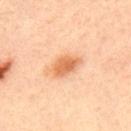Notes:
* workup: imaged on a skin check; not biopsied
* patient: male, aged 33–37
* image source: ~15 mm tile from a whole-body skin photo
* lighting: cross-polarized illumination
* lesion diameter: ≈4 mm
* site: the upper back
* image-analysis metrics: an average lesion color of about L≈66 a*≈26 b*≈40 (CIELAB), roughly 12 lightness units darker than nearby skin, and a lesion-to-skin contrast of about 8 (normalized; higher = more distinct); a border-irregularity index near 2.5/10, a color-variation rating of about 3/10, and peripheral color asymmetry of about 1; an automated nevus-likeness rating near 95 out of 100 and lesion-presence confidence of about 100/100